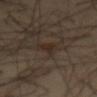Clinical impression:
The lesion was photographed on a routine skin check and not biopsied; there is no pathology result.
Acquisition and patient details:
A male patient, roughly 40 years of age. Captured under cross-polarized illumination. A close-up tile cropped from a whole-body skin photograph, about 15 mm across. Measured at roughly 3.5 mm in maximum diameter. On the abdomen.